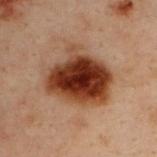Captured during whole-body skin photography for melanoma surveillance; the lesion was not biopsied.
A 15 mm close-up extracted from a 3D total-body photography capture.
A male patient, aged approximately 30.
The lesion is on the upper back.
Longest diameter approximately 7 mm.